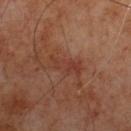Q: Was a biopsy performed?
A: catalogued during a skin exam; not biopsied